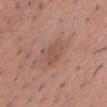subject: male, about 30 years old | lesion diameter: ≈3.5 mm | lighting: white-light illumination | automated metrics: a footprint of about 7 mm² and an eccentricity of roughly 0.7; an average lesion color of about L≈52 a*≈19 b*≈28 (CIELAB), a lesion–skin lightness drop of about 7, and a lesion-to-skin contrast of about 5 (normalized; higher = more distinct); a classifier nevus-likeness of about 0/100 and a lesion-detection confidence of about 100/100 | site: the chest | image: 15 mm crop, total-body photography.